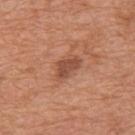Q: Was a biopsy performed?
A: total-body-photography surveillance lesion; no biopsy
Q: How large is the lesion?
A: ~3.5 mm (longest diameter)
Q: Where on the body is the lesion?
A: the mid back
Q: What is the imaging modality?
A: ~15 mm tile from a whole-body skin photo
Q: Who is the patient?
A: male, aged 63–67
Q: How was the tile lit?
A: white-light